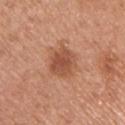Recorded during total-body skin imaging; not selected for excision or biopsy. Located on the arm. A female subject, about 65 years old. Cropped from a total-body skin-imaging series; the visible field is about 15 mm.